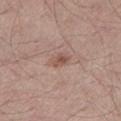  biopsy_status: not biopsied; imaged during a skin examination
  site: left lower leg
  image:
    source: total-body photography crop
    field_of_view_mm: 15
  lighting: white-light
  automated_metrics:
    eccentricity: 0.8
    shape_asymmetry: 0.2
    vs_skin_darker_L: 9.0
    color_variation_0_10: 3.0
    peripheral_color_asymmetry: 1.0
    lesion_detection_confidence_0_100: 100
  patient:
    sex: male
    age_approx: 35